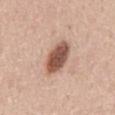follow-up: no biopsy performed (imaged during a skin exam); acquisition: ~15 mm crop, total-body skin-cancer survey; size: about 4.5 mm; lighting: white-light; location: the mid back; patient: male, aged approximately 65.